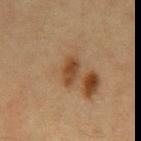Impression: Part of a total-body skin-imaging series; this lesion was reviewed on a skin check and was not flagged for biopsy. Acquisition and patient details: A male subject in their mid-60s. Located on the mid back. This image is a 15 mm lesion crop taken from a total-body photograph.